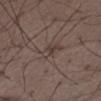biopsy status=total-body-photography surveillance lesion; no biopsy | acquisition=total-body-photography crop, ~15 mm field of view | body site=the leg | lighting=white-light | patient=male, aged approximately 50.